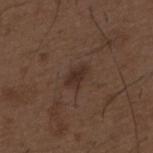<lesion>
<biopsy_status>not biopsied; imaged during a skin examination</biopsy_status>
<lighting>white-light</lighting>
<automated_metrics>
  <cielab_L>29</cielab_L>
  <cielab_a>16</cielab_a>
  <cielab_b>22</cielab_b>
  <vs_skin_darker_L>7.0</vs_skin_darker_L>
  <border_irregularity_0_10>3.0</border_irregularity_0_10>
  <color_variation_0_10>1.5</color_variation_0_10>
  <nevus_likeness_0_100>75</nevus_likeness_0_100>
  <lesion_detection_confidence_0_100>100</lesion_detection_confidence_0_100>
</automated_metrics>
<image>
  <source>total-body photography crop</source>
  <field_of_view_mm>15</field_of_view_mm>
</image>
<patient>
  <sex>male</sex>
  <age_approx>50</age_approx>
</patient>
<site>upper back</site>
</lesion>